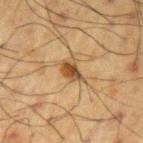{"biopsy_status": "not biopsied; imaged during a skin examination", "image": {"source": "total-body photography crop", "field_of_view_mm": 15}, "patient": {"sex": "male", "age_approx": 60}, "site": "arm", "lesion_size": {"long_diameter_mm_approx": 3.0}, "lighting": "cross-polarized"}